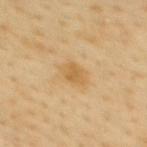| feature | finding |
|---|---|
| biopsy status | imaged on a skin check; not biopsied |
| site | the upper back |
| patient | female, aged around 50 |
| lesion size | ≈3 mm |
| image-analysis metrics | an area of roughly 5 mm² and an eccentricity of roughly 0.7; a lesion color around L≈60 a*≈17 b*≈43 in CIELAB and a lesion-to-skin contrast of about 6.5 (normalized; higher = more distinct); a border-irregularity index near 1.5/10, internal color variation of about 2 on a 0–10 scale, and peripheral color asymmetry of about 0.5; an automated nevus-likeness rating near 5 out of 100 and lesion-presence confidence of about 100/100 |
| image | 15 mm crop, total-body photography |
| tile lighting | cross-polarized illumination |